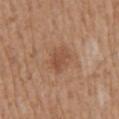notes: no biopsy performed (imaged during a skin exam)
tile lighting: white-light illumination
automated lesion analysis: an area of roughly 5 mm², a shape eccentricity near 0.75, and a shape-asymmetry score of about 0.3 (0 = symmetric); an average lesion color of about L≈50 a*≈22 b*≈32 (CIELAB), roughly 8 lightness units darker than nearby skin, and a lesion-to-skin contrast of about 6 (normalized; higher = more distinct); a border-irregularity index near 3/10, internal color variation of about 2.5 on a 0–10 scale, and peripheral color asymmetry of about 1
anatomic site: the mid back
diameter: ≈3 mm
subject: male, aged 68 to 72
image source: 15 mm crop, total-body photography